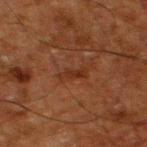Imaged during a routine full-body skin examination; the lesion was not biopsied and no histopathology is available.